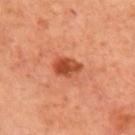Q: Was this lesion biopsied?
A: no biopsy performed (imaged during a skin exam)
Q: What is the anatomic site?
A: the chest
Q: Lesion size?
A: ≈3.5 mm
Q: How was this image acquired?
A: ~15 mm crop, total-body skin-cancer survey
Q: Illumination type?
A: cross-polarized illumination
Q: Patient demographics?
A: female, aged 53 to 57